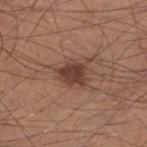Impression: The lesion was tiled from a total-body skin photograph and was not biopsied. Background: A 15 mm close-up extracted from a 3D total-body photography capture. A male subject aged approximately 30. Located on the left lower leg.